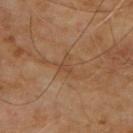The lesion was tiled from a total-body skin photograph and was not biopsied.
This is a cross-polarized tile.
A male subject aged 68–72.
This image is a 15 mm lesion crop taken from a total-body photograph.
The lesion is on the upper back.
The lesion-visualizer software estimated two-axis asymmetry of about 0.6. The analysis additionally found an average lesion color of about L≈45 a*≈19 b*≈32 (CIELAB) and a lesion-to-skin contrast of about 4.5 (normalized; higher = more distinct). The software also gave a border-irregularity rating of about 7/10, a color-variation rating of about 1.5/10, and a peripheral color-asymmetry measure near 0.5.
Measured at roughly 3 mm in maximum diameter.